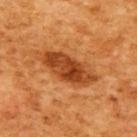Notes:
• biopsy status: imaged on a skin check; not biopsied
• image source: 15 mm crop, total-body photography
• body site: the right upper arm
• patient: female, approximately 55 years of age
• diameter: about 7 mm